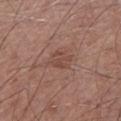Part of a total-body skin-imaging series; this lesion was reviewed on a skin check and was not flagged for biopsy. A lesion tile, about 15 mm wide, cut from a 3D total-body photograph. A male subject, aged 58 to 62. Imaged with white-light lighting. Longest diameter approximately 2.5 mm. From the left lower leg.